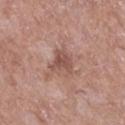Captured during whole-body skin photography for melanoma surveillance; the lesion was not biopsied. On the right lower leg. Automated image analysis of the tile measured a shape eccentricity near 0.3 and two-axis asymmetry of about 0.35. The analysis additionally found a mean CIELAB color near L≈52 a*≈21 b*≈25, a lesion–skin lightness drop of about 9, and a normalized border contrast of about 6.5. The software also gave a nevus-likeness score of about 0/100 and lesion-presence confidence of about 100/100. The patient is a female about 75 years old. The tile uses white-light illumination. A close-up tile cropped from a whole-body skin photograph, about 15 mm across. Longest diameter approximately 3 mm.